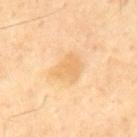Clinical impression: This lesion was catalogued during total-body skin photography and was not selected for biopsy. Background: The lesion is on the back. Automated image analysis of the tile measured an area of roughly 8.5 mm², an eccentricity of roughly 0.65, and a symmetry-axis asymmetry near 0.3. It also reported a border-irregularity rating of about 3/10 and radial color variation of about 1. And it measured a classifier nevus-likeness of about 0/100 and a lesion-detection confidence of about 100/100. A male subject aged 68 to 72. This image is a 15 mm lesion crop taken from a total-body photograph.